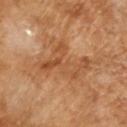follow-up: catalogued during a skin exam; not biopsied | imaging modality: ~15 mm crop, total-body skin-cancer survey | patient: male, aged 63–67.